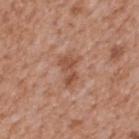Findings:
• biopsy status · total-body-photography surveillance lesion; no biopsy
• acquisition · ~15 mm tile from a whole-body skin photo
• patient · male, roughly 65 years of age
• location · the mid back